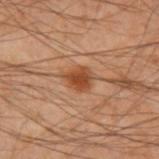Clinical summary:
The lesion is located on the left forearm. The lesion's longest dimension is about 2.5 mm. A male subject aged approximately 50. A region of skin cropped from a whole-body photographic capture, roughly 15 mm wide.